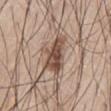{"biopsy_status": "not biopsied; imaged during a skin examination"}